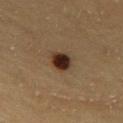biopsy_status: not biopsied; imaged during a skin examination
site: upper back
image:
  source: total-body photography crop
  field_of_view_mm: 15
patient:
  sex: female
  age_approx: 30